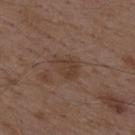The patient is a male aged around 50. An algorithmic analysis of the crop reported a color-variation rating of about 2.5/10 and radial color variation of about 1. And it measured a classifier nevus-likeness of about 0/100 and lesion-presence confidence of about 100/100. Longest diameter approximately 3 mm. This is a white-light tile. The lesion is located on the mid back. This image is a 15 mm lesion crop taken from a total-body photograph.